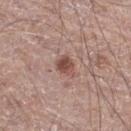Part of a total-body skin-imaging series; this lesion was reviewed on a skin check and was not flagged for biopsy. A 15 mm close-up extracted from a 3D total-body photography capture. Captured under white-light illumination. A male patient in their 70s. Automated tile analysis of the lesion measured an average lesion color of about L≈49 a*≈21 b*≈24 (CIELAB) and a normalized lesion–skin contrast near 8. The analysis additionally found a border-irregularity rating of about 2.5/10, internal color variation of about 3.5 on a 0–10 scale, and peripheral color asymmetry of about 1.5. The analysis additionally found a nevus-likeness score of about 90/100 and a lesion-detection confidence of about 100/100. From the right thigh. Longest diameter approximately 2.5 mm.